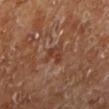Notes:
- workup — imaged on a skin check; not biopsied
- subject — male, aged around 70
- size — about 2.5 mm
- TBP lesion metrics — a footprint of about 4 mm², a shape eccentricity near 0.7, and two-axis asymmetry of about 0.4; an average lesion color of about L≈38 a*≈22 b*≈29 (CIELAB), a lesion–skin lightness drop of about 7, and a normalized lesion–skin contrast near 6.5; a color-variation rating of about 3/10 and a peripheral color-asymmetry measure near 1; a nevus-likeness score of about 0/100 and lesion-presence confidence of about 100/100
- imaging modality — ~15 mm crop, total-body skin-cancer survey
- lighting — cross-polarized
- site — the left lower leg